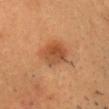Case summary:
– follow-up: no biopsy performed (imaged during a skin exam)
– patient: male, about 40 years old
– acquisition: ~15 mm tile from a whole-body skin photo
– location: the head or neck